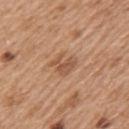* notes: no biopsy performed (imaged during a skin exam)
* subject: female, approximately 40 years of age
* imaging modality: ~15 mm tile from a whole-body skin photo
* body site: the left upper arm